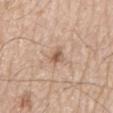Assessment:
Recorded during total-body skin imaging; not selected for excision or biopsy.
Background:
The subject is a male approximately 80 years of age. The tile uses white-light illumination. The recorded lesion diameter is about 2.5 mm. A 15 mm close-up tile from a total-body photography series done for melanoma screening. From the mid back.